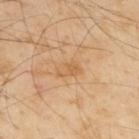Case summary:
– follow-up: imaged on a skin check; not biopsied
– location: the upper back
– image source: ~15 mm crop, total-body skin-cancer survey
– lesion size: about 3 mm
– subject: male, in their mid- to late 50s
– automated lesion analysis: an area of roughly 3.5 mm², a shape eccentricity near 0.8, and a shape-asymmetry score of about 0.4 (0 = symmetric); an average lesion color of about L≈61 a*≈20 b*≈41 (CIELAB) and roughly 7 lightness units darker than nearby skin; a border-irregularity index near 4/10 and radial color variation of about 0.5; a classifier nevus-likeness of about 0/100 and a detector confidence of about 100 out of 100 that the crop contains a lesion